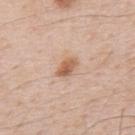Impression: The lesion was tiled from a total-body skin photograph and was not biopsied. Image and clinical context: Cropped from a total-body skin-imaging series; the visible field is about 15 mm. On the upper back. The patient is a male aged 48–52. Captured under white-light illumination.